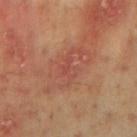Q: Is there a histopathology result?
A: no biopsy performed (imaged during a skin exam)
Q: What is the lesion's diameter?
A: about 6.5 mm
Q: What is the anatomic site?
A: the mid back
Q: What are the patient's age and sex?
A: male, in their mid- to late 50s
Q: What lighting was used for the tile?
A: cross-polarized
Q: How was this image acquired?
A: ~15 mm tile from a whole-body skin photo